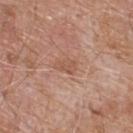Case summary:
• notes — total-body-photography surveillance lesion; no biopsy
• automated metrics — roughly 7 lightness units darker than nearby skin and a normalized border contrast of about 5.5; an automated nevus-likeness rating near 0 out of 100
• tile lighting — white-light
• site — the upper back
• acquisition — ~15 mm tile from a whole-body skin photo
• subject — male, in their 80s
• diameter — ≈3 mm